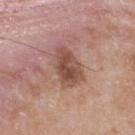On the front of the torso. The subject is a male in their 50s. Automated image analysis of the tile measured a footprint of about 12 mm², an eccentricity of roughly 0.85, and two-axis asymmetry of about 0.25. The analysis additionally found an automated nevus-likeness rating near 60 out of 100 and a lesion-detection confidence of about 100/100. Approximately 5 mm at its widest. A roughly 15 mm field-of-view crop from a total-body skin photograph. Captured under white-light illumination.